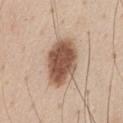Recorded during total-body skin imaging; not selected for excision or biopsy. This is a white-light tile. From the front of the torso. A roughly 15 mm field-of-view crop from a total-body skin photograph. About 6 mm across. A male patient, aged 43 to 47.